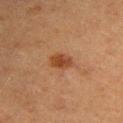This lesion was catalogued during total-body skin photography and was not selected for biopsy. A 15 mm close-up extracted from a 3D total-body photography capture. A female patient, aged approximately 55. The lesion is on the upper back. About 3 mm across. The tile uses cross-polarized illumination.